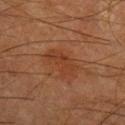notes: imaged on a skin check; not biopsied | location: the leg | patient: male, in their mid-60s | imaging modality: 15 mm crop, total-body photography.